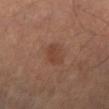From the left lower leg.
Longest diameter approximately 3 mm.
The subject is a male approximately 60 years of age.
Imaged with cross-polarized lighting.
A 15 mm crop from a total-body photograph taken for skin-cancer surveillance.